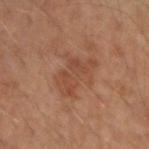Captured during whole-body skin photography for melanoma surveillance; the lesion was not biopsied.
This is a cross-polarized tile.
Located on the right forearm.
Automated tile analysis of the lesion measured a mean CIELAB color near L≈46 a*≈22 b*≈31, roughly 7 lightness units darker than nearby skin, and a normalized lesion–skin contrast near 5.5. And it measured border irregularity of about 6 on a 0–10 scale, a color-variation rating of about 2.5/10, and a peripheral color-asymmetry measure near 0.5.
The patient is a male aged around 45.
The recorded lesion diameter is about 4.5 mm.
Cropped from a total-body skin-imaging series; the visible field is about 15 mm.